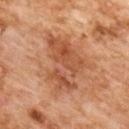Clinical impression:
Imaged during a routine full-body skin examination; the lesion was not biopsied and no histopathology is available.
Clinical summary:
A female patient aged approximately 60. A lesion tile, about 15 mm wide, cut from a 3D total-body photograph. The lesion-visualizer software estimated a footprint of about 21 mm² and a shape eccentricity near 0.8. It also reported border irregularity of about 5.5 on a 0–10 scale, a color-variation rating of about 5/10, and radial color variation of about 1.5. This is a cross-polarized tile. From the upper back. About 7 mm across.